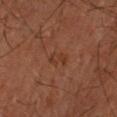{
  "patient": {
    "sex": "male",
    "age_approx": 70
  },
  "site": "right forearm",
  "image": {
    "source": "total-body photography crop",
    "field_of_view_mm": 15
  },
  "automated_metrics": {
    "vs_skin_darker_L": 4.0,
    "vs_skin_contrast_norm": 4.5,
    "nevus_likeness_0_100": 0,
    "lesion_detection_confidence_0_100": 100
  },
  "lighting": "cross-polarized",
  "lesion_size": {
    "long_diameter_mm_approx": 3.0
  }
}